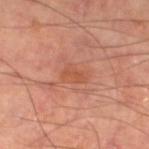Q: Was a biopsy performed?
A: total-body-photography surveillance lesion; no biopsy
Q: What is the imaging modality?
A: 15 mm crop, total-body photography
Q: What is the lesion's diameter?
A: about 2.5 mm
Q: Automated lesion metrics?
A: an area of roughly 4 mm², an eccentricity of roughly 0.75, and two-axis asymmetry of about 0.3; a lesion color around L≈53 a*≈27 b*≈34 in CIELAB, a lesion–skin lightness drop of about 6, and a lesion-to-skin contrast of about 5.5 (normalized; higher = more distinct); a border-irregularity rating of about 3/10, a within-lesion color-variation index near 1.5/10, and peripheral color asymmetry of about 0.5; a detector confidence of about 100 out of 100 that the crop contains a lesion
Q: What lighting was used for the tile?
A: cross-polarized
Q: Lesion location?
A: the leg
Q: What are the patient's age and sex?
A: male, in their mid- to late 60s